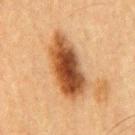Part of a total-body skin-imaging series; this lesion was reviewed on a skin check and was not flagged for biopsy. This is a cross-polarized tile. From the chest. Automated image analysis of the tile measured a lesion area of about 23 mm², an outline eccentricity of about 0.9 (0 = round, 1 = elongated), and two-axis asymmetry of about 0.15. And it measured a mean CIELAB color near L≈43 a*≈21 b*≈34, about 16 CIELAB-L* units darker than the surrounding skin, and a lesion-to-skin contrast of about 12 (normalized; higher = more distinct). And it measured a nevus-likeness score of about 95/100. Cropped from a whole-body photographic skin survey; the tile spans about 15 mm. The subject is a male roughly 65 years of age. Longest diameter approximately 8 mm.